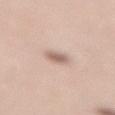– follow-up · catalogued during a skin exam; not biopsied
– size · ~2.5 mm (longest diameter)
– automated metrics · an outline eccentricity of about 0.5 (0 = round, 1 = elongated) and a shape-asymmetry score of about 0.2 (0 = symmetric); an average lesion color of about L≈63 a*≈17 b*≈25 (CIELAB) and a lesion-to-skin contrast of about 7 (normalized; higher = more distinct); a border-irregularity rating of about 2/10, a color-variation rating of about 2/10, and peripheral color asymmetry of about 0.5; a nevus-likeness score of about 90/100 and lesion-presence confidence of about 100/100
– patient · female, aged around 45
– tile lighting · white-light illumination
– location · the lower back
– image · 15 mm crop, total-body photography